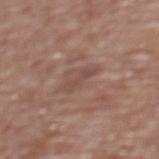Clinical impression: The lesion was photographed on a routine skin check and not biopsied; there is no pathology result. Clinical summary: About 3.5 mm across. Located on the back. The patient is a female aged 78 to 82. Imaged with white-light lighting. A region of skin cropped from a whole-body photographic capture, roughly 15 mm wide.